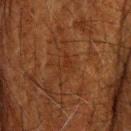{"biopsy_status": "not biopsied; imaged during a skin examination", "lesion_size": {"long_diameter_mm_approx": 3.0}, "lighting": "cross-polarized", "patient": {"sex": "male", "age_approx": 60}, "image": {"source": "total-body photography crop", "field_of_view_mm": 15}, "site": "head or neck"}